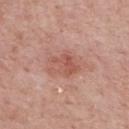Imaged during a routine full-body skin examination; the lesion was not biopsied and no histopathology is available. A region of skin cropped from a whole-body photographic capture, roughly 15 mm wide. A male patient in their mid- to late 60s. The lesion is located on the mid back. The tile uses white-light illumination. About 4 mm across.